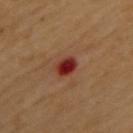The lesion is on the upper back.
The recorded lesion diameter is about 2.5 mm.
The subject is a female roughly 60 years of age.
Captured under cross-polarized illumination.
A region of skin cropped from a whole-body photographic capture, roughly 15 mm wide.